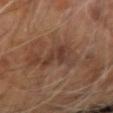The lesion was tiled from a total-body skin photograph and was not biopsied.